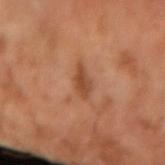The lesion was photographed on a routine skin check and not biopsied; there is no pathology result.
Cropped from a whole-body photographic skin survey; the tile spans about 15 mm.
A male patient in their mid-60s.